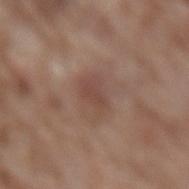<tbp_lesion>
<biopsy_status>not biopsied; imaged during a skin examination</biopsy_status>
<image>
  <source>total-body photography crop</source>
  <field_of_view_mm>15</field_of_view_mm>
</image>
<lighting>white-light</lighting>
<lesion_size>
  <long_diameter_mm_approx>3.5</long_diameter_mm_approx>
</lesion_size>
<site>lower back</site>
<patient>
  <sex>male</sex>
  <age_approx>75</age_approx>
</patient>
</tbp_lesion>